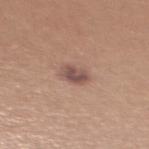Q: What did automated image analysis measure?
A: an outline eccentricity of about 0.75 (0 = round, 1 = elongated); a normalized lesion–skin contrast near 8.5; a lesion-detection confidence of about 100/100
Q: How large is the lesion?
A: ≈3 mm
Q: What kind of image is this?
A: 15 mm crop, total-body photography
Q: Where on the body is the lesion?
A: the upper back
Q: Patient demographics?
A: female, aged approximately 40